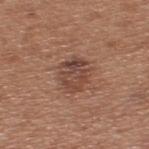| feature | finding |
|---|---|
| biopsy status | total-body-photography surveillance lesion; no biopsy |
| TBP lesion metrics | a lesion color around L≈45 a*≈21 b*≈26 in CIELAB, a lesion–skin lightness drop of about 9, and a normalized border contrast of about 7 |
| tile lighting | white-light |
| acquisition | ~15 mm tile from a whole-body skin photo |
| subject | male, in their mid-50s |
| body site | the upper back |